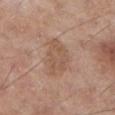notes: imaged on a skin check; not biopsied
subject: male, aged approximately 55
size: ~5 mm (longest diameter)
lighting: white-light
image-analysis metrics: a footprint of about 10 mm², a shape eccentricity near 0.8, and a shape-asymmetry score of about 0.3 (0 = symmetric); border irregularity of about 3.5 on a 0–10 scale and a peripheral color-asymmetry measure near 1; a nevus-likeness score of about 0/100 and a lesion-detection confidence of about 100/100
body site: the left lower leg
image source: ~15 mm crop, total-body skin-cancer survey